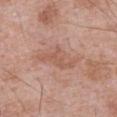Image and clinical context:
Captured under white-light illumination. A male patient approximately 70 years of age. Automated tile analysis of the lesion measured an area of roughly 10 mm² and a shape eccentricity near 0.8. It also reported border irregularity of about 4.5 on a 0–10 scale, a within-lesion color-variation index near 2.5/10, and radial color variation of about 1. Approximately 5 mm at its widest. A 15 mm close-up tile from a total-body photography series done for melanoma screening. From the abdomen.